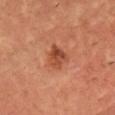This is a cross-polarized tile.
A 15 mm crop from a total-body photograph taken for skin-cancer surveillance.
From the head or neck.
Longest diameter approximately 3 mm.
A male patient roughly 55 years of age.